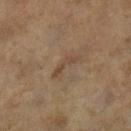The lesion was tiled from a total-body skin photograph and was not biopsied.
The recorded lesion diameter is about 4 mm.
The tile uses cross-polarized illumination.
A female subject about 60 years old.
A 15 mm close-up extracted from a 3D total-body photography capture.
The lesion is on the leg.